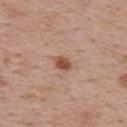Q: Was a biopsy performed?
A: total-body-photography surveillance lesion; no biopsy
Q: What did automated image analysis measure?
A: a lesion color around L≈52 a*≈21 b*≈30 in CIELAB and roughly 12 lightness units darker than nearby skin; a nevus-likeness score of about 95/100
Q: Patient demographics?
A: male, roughly 70 years of age
Q: What kind of image is this?
A: ~15 mm tile from a whole-body skin photo
Q: How large is the lesion?
A: ≈2.5 mm
Q: What is the anatomic site?
A: the upper back
Q: How was the tile lit?
A: white-light illumination